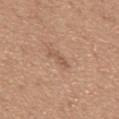<tbp_lesion>
<biopsy_status>not biopsied; imaged during a skin examination</biopsy_status>
<patient>
  <sex>male</sex>
  <age_approx>65</age_approx>
</patient>
<site>mid back</site>
<image>
  <source>total-body photography crop</source>
  <field_of_view_mm>15</field_of_view_mm>
</image>
</tbp_lesion>